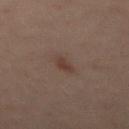– lighting — cross-polarized
– site — the chest
– lesion diameter — about 2.5 mm
– patient — male, approximately 40 years of age
– imaging modality — 15 mm crop, total-body photography